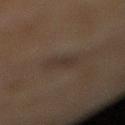lesion size = ≈3 mm
anatomic site = the right lower leg
lighting = cross-polarized
image-analysis metrics = a lesion area of about 3.5 mm² and a shape eccentricity near 0.85; a classifier nevus-likeness of about 10/100 and a lesion-detection confidence of about 95/100
patient = female, in their 60s
imaging modality = ~15 mm tile from a whole-body skin photo